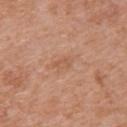Q: Is there a histopathology result?
A: no biopsy performed (imaged during a skin exam)
Q: What is the anatomic site?
A: the upper back
Q: Automated lesion metrics?
A: about 6 CIELAB-L* units darker than the surrounding skin and a normalized lesion–skin contrast near 4.5; a detector confidence of about 100 out of 100 that the crop contains a lesion
Q: What are the patient's age and sex?
A: female, in their 40s
Q: What is the imaging modality?
A: ~15 mm crop, total-body skin-cancer survey
Q: Lesion size?
A: about 2.5 mm